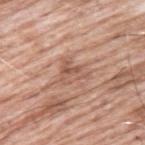Recorded during total-body skin imaging; not selected for excision or biopsy.
A male subject in their 60s.
Captured under white-light illumination.
Cropped from a whole-body photographic skin survey; the tile spans about 15 mm.
Located on the back.